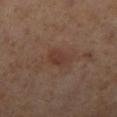follow-up: imaged on a skin check; not biopsied | lesion diameter: ~2.5 mm (longest diameter) | patient: female, roughly 55 years of age | anatomic site: the leg | image: total-body-photography crop, ~15 mm field of view | tile lighting: cross-polarized | automated lesion analysis: an eccentricity of roughly 0.75 and two-axis asymmetry of about 0.25; a lesion color around L≈36 a*≈20 b*≈25 in CIELAB and about 6 CIELAB-L* units darker than the surrounding skin; a lesion-detection confidence of about 100/100.